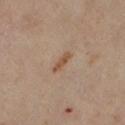| field | value |
|---|---|
| notes | catalogued during a skin exam; not biopsied |
| location | the left lower leg |
| subject | female, in their 60s |
| illumination | cross-polarized illumination |
| diameter | about 3 mm |
| image source | total-body-photography crop, ~15 mm field of view |
| automated lesion analysis | a lesion area of about 3 mm², an outline eccentricity of about 0.95 (0 = round, 1 = elongated), and a symmetry-axis asymmetry near 0.25; about 8 CIELAB-L* units darker than the surrounding skin and a normalized lesion–skin contrast near 7 |